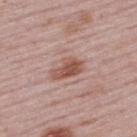No biopsy was performed on this lesion — it was imaged during a full skin examination and was not determined to be concerning. The lesion is located on the upper back. Automated tile analysis of the lesion measured roughly 11 lightness units darker than nearby skin and a lesion-to-skin contrast of about 8.5 (normalized; higher = more distinct). And it measured border irregularity of about 3.5 on a 0–10 scale, a within-lesion color-variation index near 3.5/10, and radial color variation of about 1.5. It also reported a nevus-likeness score of about 20/100 and lesion-presence confidence of about 100/100. A female subject, roughly 50 years of age. Cropped from a whole-body photographic skin survey; the tile spans about 15 mm. Imaged with white-light lighting.